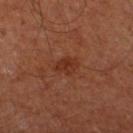- notes · imaged on a skin check; not biopsied
- acquisition · ~15 mm tile from a whole-body skin photo
- tile lighting · cross-polarized illumination
- image-analysis metrics · a border-irregularity index near 2/10, a color-variation rating of about 2.5/10, and a peripheral color-asymmetry measure near 1
- size · about 3 mm
- location · the right lower leg
- patient · male, roughly 65 years of age